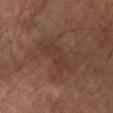Clinical impression:
Recorded during total-body skin imaging; not selected for excision or biopsy.
Background:
The lesion is on the left lower leg. Measured at roughly 6 mm in maximum diameter. The patient is a male approximately 65 years of age. A close-up tile cropped from a whole-body skin photograph, about 15 mm across. Captured under cross-polarized illumination.